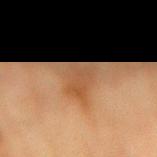Assessment: The lesion was photographed on a routine skin check and not biopsied; there is no pathology result. Context: On the mid back. A female patient approximately 80 years of age. Imaged with cross-polarized lighting. Longest diameter approximately 5 mm. A roughly 15 mm field-of-view crop from a total-body skin photograph.